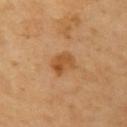No biopsy was performed on this lesion — it was imaged during a full skin examination and was not determined to be concerning.
Captured under cross-polarized illumination.
An algorithmic analysis of the crop reported a lesion area of about 5.5 mm², a shape eccentricity near 0.75, and a symmetry-axis asymmetry near 0.4. And it measured a lesion–skin lightness drop of about 10 and a normalized border contrast of about 7.5. The software also gave a classifier nevus-likeness of about 45/100 and a detector confidence of about 100 out of 100 that the crop contains a lesion.
A roughly 15 mm field-of-view crop from a total-body skin photograph.
Approximately 3 mm at its widest.
A subject aged approximately 60.
Located on the left upper arm.